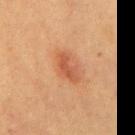<record>
<biopsy_status>not biopsied; imaged during a skin examination</biopsy_status>
<site>back</site>
<automated_metrics>
  <area_mm2_approx>5.0</area_mm2_approx>
  <eccentricity>0.9</eccentricity>
  <shape_asymmetry>0.35</shape_asymmetry>
  <cielab_L>49</cielab_L>
  <cielab_a>25</cielab_a>
  <cielab_b>33</cielab_b>
  <vs_skin_contrast_norm>6.5</vs_skin_contrast_norm>
  <color_variation_0_10>2.5</color_variation_0_10>
  <peripheral_color_asymmetry>1.0</peripheral_color_asymmetry>
</automated_metrics>
<lighting>cross-polarized</lighting>
<patient>
  <sex>female</sex>
  <age_approx>60</age_approx>
</patient>
<lesion_size>
  <long_diameter_mm_approx>3.5</long_diameter_mm_approx>
</lesion_size>
<image>
  <source>total-body photography crop</source>
  <field_of_view_mm>15</field_of_view_mm>
</image>
</record>